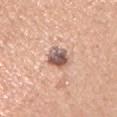The lesion is on the arm.
A male patient about 45 years old.
Approximately 3.5 mm at its widest.
Cropped from a whole-body photographic skin survey; the tile spans about 15 mm.